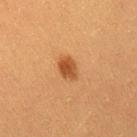notes: total-body-photography surveillance lesion; no biopsy
patient: female, aged approximately 40
imaging modality: ~15 mm crop, total-body skin-cancer survey
automated lesion analysis: a lesion area of about 5 mm², an eccentricity of roughly 0.7, and two-axis asymmetry of about 0.2; an average lesion color of about L≈41 a*≈23 b*≈35 (CIELAB), roughly 10 lightness units darker than nearby skin, and a lesion-to-skin contrast of about 8.5 (normalized; higher = more distinct); border irregularity of about 2 on a 0–10 scale; a lesion-detection confidence of about 100/100
anatomic site: the leg